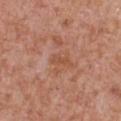Q: Is there a histopathology result?
A: imaged on a skin check; not biopsied
Q: Who is the patient?
A: male, in their mid-60s
Q: Where on the body is the lesion?
A: the chest
Q: What is the imaging modality?
A: total-body-photography crop, ~15 mm field of view